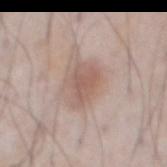Q: Is there a histopathology result?
A: no biopsy performed (imaged during a skin exam)
Q: What is the lesion's diameter?
A: about 4.5 mm
Q: What did automated image analysis measure?
A: a mean CIELAB color near L≈58 a*≈18 b*≈24, about 9 CIELAB-L* units darker than the surrounding skin, and a normalized border contrast of about 6; a nevus-likeness score of about 70/100 and lesion-presence confidence of about 100/100
Q: Where on the body is the lesion?
A: the abdomen
Q: What lighting was used for the tile?
A: white-light illumination
Q: Patient demographics?
A: male, aged 73–77
Q: What is the imaging modality?
A: total-body-photography crop, ~15 mm field of view